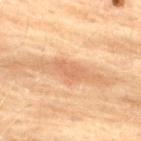The lesion was tiled from a total-body skin photograph and was not biopsied. The lesion is on the upper back. The subject is a female about 80 years old. Cropped from a total-body skin-imaging series; the visible field is about 15 mm. The tile uses cross-polarized illumination.